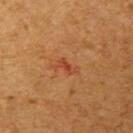Imaged during a routine full-body skin examination; the lesion was not biopsied and no histopathology is available. This image is a 15 mm lesion crop taken from a total-body photograph. On the arm. A male patient roughly 45 years of age.